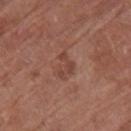Assessment:
Imaged during a routine full-body skin examination; the lesion was not biopsied and no histopathology is available.
Context:
This is a white-light tile. The lesion-visualizer software estimated a lesion area of about 3.5 mm² and a shape-asymmetry score of about 0.7 (0 = symmetric). And it measured an average lesion color of about L≈43 a*≈23 b*≈27 (CIELAB), roughly 7 lightness units darker than nearby skin, and a normalized border contrast of about 6. It also reported a border-irregularity rating of about 8/10, a within-lesion color-variation index near 0/10, and a peripheral color-asymmetry measure near 0. The recorded lesion diameter is about 3 mm. A region of skin cropped from a whole-body photographic capture, roughly 15 mm wide. A female patient, roughly 80 years of age. Located on the right thigh.